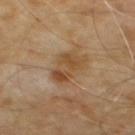Captured during whole-body skin photography for melanoma surveillance; the lesion was not biopsied. Captured under cross-polarized illumination. About 5 mm across. A region of skin cropped from a whole-body photographic capture, roughly 15 mm wide. A male patient aged 58 to 62. The lesion is on the chest. An algorithmic analysis of the crop reported a footprint of about 9 mm², a shape eccentricity near 0.85, and a symmetry-axis asymmetry near 0.25. The software also gave a within-lesion color-variation index near 5/10 and peripheral color asymmetry of about 1.5. It also reported a nevus-likeness score of about 0/100 and lesion-presence confidence of about 100/100.